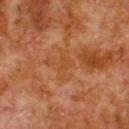No biopsy was performed on this lesion — it was imaged during a full skin examination and was not determined to be concerning. About 3.5 mm across. Imaged with cross-polarized lighting. Automated tile analysis of the lesion measured roughly 4 lightness units darker than nearby skin and a normalized border contrast of about 4.5. And it measured a border-irregularity index near 4.5/10 and peripheral color asymmetry of about 0.5. A male patient in their 80s. From the upper back. This image is a 15 mm lesion crop taken from a total-body photograph.